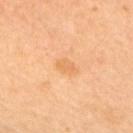biopsy_status: not biopsied; imaged during a skin examination
site: upper back
lesion_size:
  long_diameter_mm_approx: 3.0
patient:
  sex: female
  age_approx: 50
lighting: cross-polarized
image:
  source: total-body photography crop
  field_of_view_mm: 15